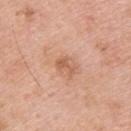Clinical summary:
About 2.5 mm across. A close-up tile cropped from a whole-body skin photograph, about 15 mm across. Imaged with white-light lighting. A male subject, aged around 60. An algorithmic analysis of the crop reported an average lesion color of about L≈59 a*≈24 b*≈34 (CIELAB), roughly 9 lightness units darker than nearby skin, and a normalized border contrast of about 6. The analysis additionally found a border-irregularity rating of about 5.5/10 and a peripheral color-asymmetry measure near 0. The lesion is on the upper back.